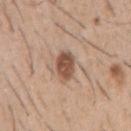Impression:
The lesion was photographed on a routine skin check and not biopsied; there is no pathology result.
Image and clinical context:
The lesion is located on the right upper arm. A 15 mm close-up extracted from a 3D total-body photography capture. About 3.5 mm across. A male patient, approximately 50 years of age.